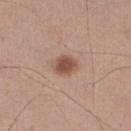The lesion is located on the right lower leg. A region of skin cropped from a whole-body photographic capture, roughly 15 mm wide. A male patient aged approximately 50.